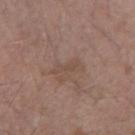Impression:
The lesion was photographed on a routine skin check and not biopsied; there is no pathology result.
Acquisition and patient details:
Located on the arm. A male subject in their 60s. A roughly 15 mm field-of-view crop from a total-body skin photograph.